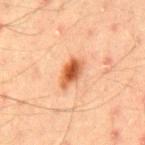{"lesion_size": {"long_diameter_mm_approx": 4.0}, "lighting": "cross-polarized", "patient": {"sex": "male", "age_approx": 45}, "site": "mid back", "image": {"source": "total-body photography crop", "field_of_view_mm": 15}}